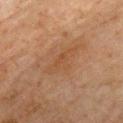Q: Was this lesion biopsied?
A: no biopsy performed (imaged during a skin exam)